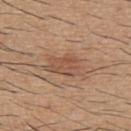follow-up: total-body-photography surveillance lesion; no biopsy
illumination: white-light illumination
TBP lesion metrics: a footprint of about 10 mm², an eccentricity of roughly 0.85, and a symmetry-axis asymmetry near 0.25; a lesion color around L≈52 a*≈20 b*≈30 in CIELAB and a normalized lesion–skin contrast near 6; a border-irregularity rating of about 3/10, a color-variation rating of about 3/10, and peripheral color asymmetry of about 1; a lesion-detection confidence of about 100/100
acquisition: total-body-photography crop, ~15 mm field of view
patient: male, in their 60s
body site: the upper back
lesion diameter: about 5 mm